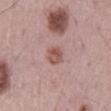Clinical impression: This lesion was catalogued during total-body skin photography and was not selected for biopsy. Background: A close-up tile cropped from a whole-body skin photograph, about 15 mm across. A male subject, aged approximately 70. The lesion is on the mid back. Captured under white-light illumination. The lesion's longest dimension is about 3 mm.